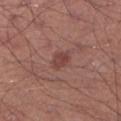Imaged during a routine full-body skin examination; the lesion was not biopsied and no histopathology is available. A male patient approximately 45 years of age. A lesion tile, about 15 mm wide, cut from a 3D total-body photograph. About 3 mm across. The lesion is located on the right lower leg. Imaged with white-light lighting.